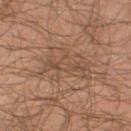<tbp_lesion>
  <biopsy_status>not biopsied; imaged during a skin examination</biopsy_status>
  <image>
    <source>total-body photography crop</source>
    <field_of_view_mm>15</field_of_view_mm>
  </image>
  <lighting>cross-polarized</lighting>
  <site>right thigh</site>
  <lesion_size>
    <long_diameter_mm_approx>6.0</long_diameter_mm_approx>
  </lesion_size>
  <patient>
    <sex>male</sex>
    <age_approx>65</age_approx>
  </patient>
</tbp_lesion>